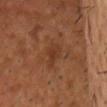{"biopsy_status": "not biopsied; imaged during a skin examination", "patient": {"sex": "male", "age_approx": 55}, "site": "head or neck", "lighting": "cross-polarized", "image": {"source": "total-body photography crop", "field_of_view_mm": 15}, "lesion_size": {"long_diameter_mm_approx": 3.0}}